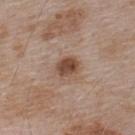<tbp_lesion>
<biopsy_status>not biopsied; imaged during a skin examination</biopsy_status>
<patient>
  <sex>male</sex>
  <age_approx>55</age_approx>
</patient>
<image>
  <source>total-body photography crop</source>
  <field_of_view_mm>15</field_of_view_mm>
</image>
<lighting>white-light</lighting>
<automated_metrics>
  <vs_skin_darker_L>13.0</vs_skin_darker_L>
  <vs_skin_contrast_norm>9.5</vs_skin_contrast_norm>
</automated_metrics>
<site>upper back</site>
</tbp_lesion>